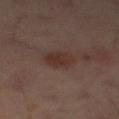Clinical impression: The lesion was tiled from a total-body skin photograph and was not biopsied. Context: This is a cross-polarized tile. The lesion's longest dimension is about 3.5 mm. The lesion is located on the mid back. A region of skin cropped from a whole-body photographic capture, roughly 15 mm wide. The lesion-visualizer software estimated a footprint of about 6.5 mm², an outline eccentricity of about 0.7 (0 = round, 1 = elongated), and two-axis asymmetry of about 0.15. The software also gave a lesion color around L≈29 a*≈16 b*≈20 in CIELAB, roughly 6 lightness units darker than nearby skin, and a lesion-to-skin contrast of about 7 (normalized; higher = more distinct). The analysis additionally found an automated nevus-likeness rating near 50 out of 100 and lesion-presence confidence of about 100/100. A male subject in their 60s.